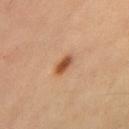A male subject, about 55 years old. Cropped from a whole-body photographic skin survey; the tile spans about 15 mm. Located on the upper back. The lesion's longest dimension is about 3 mm.